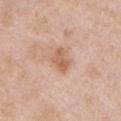biopsy_status: not biopsied; imaged during a skin examination
lighting: white-light
site: left upper arm
patient:
  sex: male
  age_approx: 50
lesion_size:
  long_diameter_mm_approx: 3.0
image:
  source: total-body photography crop
  field_of_view_mm: 15